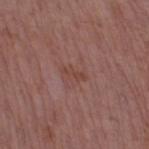Part of a total-body skin-imaging series; this lesion was reviewed on a skin check and was not flagged for biopsy. From the right thigh. A lesion tile, about 15 mm wide, cut from a 3D total-body photograph. The lesion's longest dimension is about 3 mm. A male subject, in their 70s. An algorithmic analysis of the crop reported a mean CIELAB color near L≈43 a*≈23 b*≈24, a lesion–skin lightness drop of about 6, and a normalized lesion–skin contrast near 6. And it measured a border-irregularity index near 4/10, a within-lesion color-variation index near 0/10, and a peripheral color-asymmetry measure near 0.